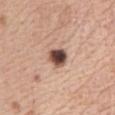Clinical impression: This lesion was catalogued during total-body skin photography and was not selected for biopsy.